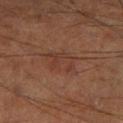Assessment: The lesion was tiled from a total-body skin photograph and was not biopsied. Context: Measured at roughly 4 mm in maximum diameter. This is a cross-polarized tile. A close-up tile cropped from a whole-body skin photograph, about 15 mm across. A male patient, in their mid- to late 60s. On the leg. The total-body-photography lesion software estimated a lesion area of about 9.5 mm² and a shape eccentricity near 0.75. It also reported an average lesion color of about L≈34 a*≈19 b*≈25 (CIELAB), a lesion–skin lightness drop of about 5, and a normalized border contrast of about 5. And it measured internal color variation of about 3 on a 0–10 scale and radial color variation of about 1. It also reported an automated nevus-likeness rating near 0 out of 100 and a detector confidence of about 100 out of 100 that the crop contains a lesion.